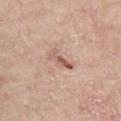A roughly 15 mm field-of-view crop from a total-body skin photograph. A male subject, about 65 years old. Approximately 3.5 mm at its widest. The lesion is on the chest. This is a white-light tile.